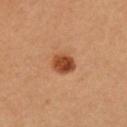Findings:
- workup · total-body-photography surveillance lesion; no biopsy
- patient · female, aged around 50
- acquisition · ~15 mm tile from a whole-body skin photo